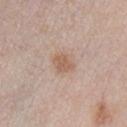Captured during whole-body skin photography for melanoma surveillance; the lesion was not biopsied. Imaged with white-light lighting. The patient is a male in their 30s. The recorded lesion diameter is about 3 mm. Cropped from a total-body skin-imaging series; the visible field is about 15 mm. The total-body-photography lesion software estimated a lesion color around L≈59 a*≈18 b*≈29 in CIELAB and a lesion-to-skin contrast of about 6.5 (normalized; higher = more distinct). The analysis additionally found a nevus-likeness score of about 65/100 and lesion-presence confidence of about 100/100.